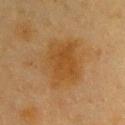No biopsy was performed on this lesion — it was imaged during a full skin examination and was not determined to be concerning. Approximately 6 mm at its widest. Cropped from a total-body skin-imaging series; the visible field is about 15 mm. The subject is a female aged 38–42. The tile uses cross-polarized illumination. The lesion is on the back.